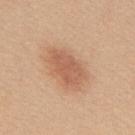Imaged during a routine full-body skin examination; the lesion was not biopsied and no histopathology is available.
A female patient approximately 45 years of age.
Located on the upper back.
A roughly 15 mm field-of-view crop from a total-body skin photograph.
Approximately 5 mm at its widest.
Captured under white-light illumination.